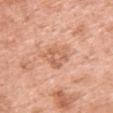Q: Is there a histopathology result?
A: catalogued during a skin exam; not biopsied
Q: What is the imaging modality?
A: 15 mm crop, total-body photography
Q: What are the patient's age and sex?
A: female, in their 50s
Q: Where on the body is the lesion?
A: the chest
Q: What did automated image analysis measure?
A: an average lesion color of about L≈63 a*≈24 b*≈34 (CIELAB) and a normalized border contrast of about 6; lesion-presence confidence of about 100/100
Q: What is the lesion's diameter?
A: about 3.5 mm